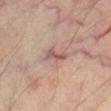<case>
  <biopsy_status>not biopsied; imaged during a skin examination</biopsy_status>
  <image>
    <source>total-body photography crop</source>
    <field_of_view_mm>15</field_of_view_mm>
  </image>
  <patient>
    <sex>male</sex>
    <age_approx>60</age_approx>
  </patient>
  <site>right thigh</site>
</case>